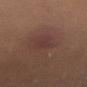Q: Was this lesion biopsied?
A: total-body-photography surveillance lesion; no biopsy
Q: What is the lesion's diameter?
A: about 3 mm
Q: How was this image acquired?
A: total-body-photography crop, ~15 mm field of view
Q: What are the patient's age and sex?
A: female, about 40 years old
Q: How was the tile lit?
A: white-light
Q: Where on the body is the lesion?
A: the right thigh